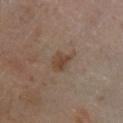Q: Patient demographics?
A: male, roughly 60 years of age
Q: What is the anatomic site?
A: the left lower leg
Q: How was this image acquired?
A: ~15 mm tile from a whole-body skin photo